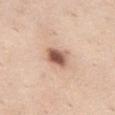{
  "biopsy_status": "not biopsied; imaged during a skin examination",
  "patient": {
    "sex": "female",
    "age_approx": 55
  },
  "automated_metrics": {
    "eccentricity": 0.65,
    "shape_asymmetry": 0.2,
    "vs_skin_darker_L": 17.0,
    "vs_skin_contrast_norm": 10.5,
    "lesion_detection_confidence_0_100": 100
  },
  "lighting": "white-light",
  "image": {
    "source": "total-body photography crop",
    "field_of_view_mm": 15
  },
  "site": "left thigh",
  "lesion_size": {
    "long_diameter_mm_approx": 3.0
  }
}